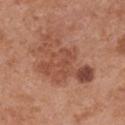{
  "lighting": "white-light",
  "image": {
    "source": "total-body photography crop",
    "field_of_view_mm": 15
  },
  "patient": {
    "sex": "female",
    "age_approx": 65
  },
  "lesion_size": {
    "long_diameter_mm_approx": 8.0
  },
  "site": "chest"
}